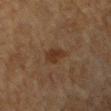Q: Is there a histopathology result?
A: no biopsy performed (imaged during a skin exam)
Q: What is the imaging modality?
A: 15 mm crop, total-body photography
Q: Automated lesion metrics?
A: a mean CIELAB color near L≈29 a*≈16 b*≈26 and a lesion-to-skin contrast of about 7.5 (normalized; higher = more distinct)
Q: Who is the patient?
A: female, approximately 55 years of age
Q: How large is the lesion?
A: about 2.5 mm
Q: What lighting was used for the tile?
A: cross-polarized
Q: Lesion location?
A: the right forearm